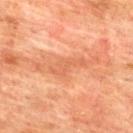Q: Was a biopsy performed?
A: imaged on a skin check; not biopsied
Q: Where on the body is the lesion?
A: the upper back
Q: What did automated image analysis measure?
A: a shape eccentricity near 0.95 and two-axis asymmetry of about 0.55; a lesion color around L≈52 a*≈25 b*≈34 in CIELAB and a lesion-to-skin contrast of about 5 (normalized; higher = more distinct); a border-irregularity rating of about 8/10, a within-lesion color-variation index near 1.5/10, and a peripheral color-asymmetry measure near 0.5
Q: How was the tile lit?
A: cross-polarized
Q: How was this image acquired?
A: 15 mm crop, total-body photography
Q: Who is the patient?
A: male, in their mid-70s